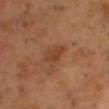The lesion was tiled from a total-body skin photograph and was not biopsied. The recorded lesion diameter is about 3 mm. This is a cross-polarized tile. Located on the chest. Cropped from a whole-body photographic skin survey; the tile spans about 15 mm. A male subject, aged around 50.